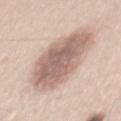notes: no biopsy performed (imaged during a skin exam)
image: ~15 mm crop, total-body skin-cancer survey
location: the mid back
patient: male, aged 43–47
lesion size: about 9 mm
illumination: white-light